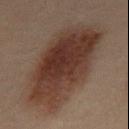workup: no biopsy performed (imaged during a skin exam)
automated lesion analysis: a footprint of about 55 mm², a shape eccentricity near 0.85, and a symmetry-axis asymmetry near 0.15; border irregularity of about 2.5 on a 0–10 scale, a within-lesion color-variation index near 5.5/10, and peripheral color asymmetry of about 2
subject: male, in their 50s
image: total-body-photography crop, ~15 mm field of view
lesion diameter: ≈11.5 mm
location: the back